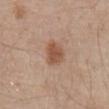<case>
<biopsy_status>not biopsied; imaged during a skin examination</biopsy_status>
<patient>
  <sex>male</sex>
  <age_approx>80</age_approx>
</patient>
<site>front of the torso</site>
<lesion_size>
  <long_diameter_mm_approx>3.0</long_diameter_mm_approx>
</lesion_size>
<image>
  <source>total-body photography crop</source>
  <field_of_view_mm>15</field_of_view_mm>
</image>
</case>